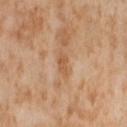Impression:
No biopsy was performed on this lesion — it was imaged during a full skin examination and was not determined to be concerning.
Background:
Cropped from a total-body skin-imaging series; the visible field is about 15 mm. A female patient in their mid-50s. Located on the right thigh. Automated image analysis of the tile measured a lesion area of about 3.5 mm² and a shape eccentricity near 0.85. And it measured a classifier nevus-likeness of about 0/100 and a detector confidence of about 100 out of 100 that the crop contains a lesion. The tile uses cross-polarized illumination.